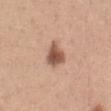This lesion was catalogued during total-body skin photography and was not selected for biopsy. A 15 mm crop from a total-body photograph taken for skin-cancer surveillance. The patient is a female approximately 30 years of age. Measured at roughly 3.5 mm in maximum diameter. The tile uses white-light illumination. The lesion is on the front of the torso. An algorithmic analysis of the crop reported a lesion area of about 7 mm², an eccentricity of roughly 0.65, and two-axis asymmetry of about 0.3. It also reported a mean CIELAB color near L≈55 a*≈21 b*≈29.